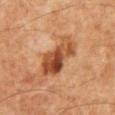Part of a total-body skin-imaging series; this lesion was reviewed on a skin check and was not flagged for biopsy.